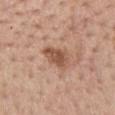workup: total-body-photography surveillance lesion; no biopsy
image source: ~15 mm tile from a whole-body skin photo
tile lighting: white-light
location: the mid back
diameter: ~4 mm (longest diameter)
subject: female, aged around 65